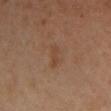<record>
<biopsy_status>not biopsied; imaged during a skin examination</biopsy_status>
<lesion_size>
  <long_diameter_mm_approx>2.5</long_diameter_mm_approx>
</lesion_size>
<automated_metrics>
  <area_mm2_approx>2.5</area_mm2_approx>
  <eccentricity>0.9</eccentricity>
</automated_metrics>
<image>
  <source>total-body photography crop</source>
  <field_of_view_mm>15</field_of_view_mm>
</image>
<site>chest</site>
<lighting>cross-polarized</lighting>
<patient>
  <sex>female</sex>
  <age_approx>35</age_approx>
</patient>
</record>